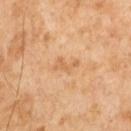{
  "biopsy_status": "not biopsied; imaged during a skin examination",
  "lesion_size": {
    "long_diameter_mm_approx": 3.0
  },
  "patient": {
    "sex": "male",
    "age_approx": 65
  },
  "automated_metrics": {
    "area_mm2_approx": 4.0,
    "eccentricity": 0.85,
    "nevus_likeness_0_100": 0,
    "lesion_detection_confidence_0_100": 100
  },
  "image": {
    "source": "total-body photography crop",
    "field_of_view_mm": 15
  },
  "lighting": "cross-polarized"
}